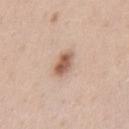biopsy_status: not biopsied; imaged during a skin examination